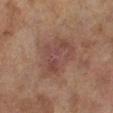Q: Was this lesion biopsied?
A: imaged on a skin check; not biopsied
Q: What is the anatomic site?
A: the left lower leg
Q: How large is the lesion?
A: ≈6.5 mm
Q: Who is the patient?
A: female, in their 60s
Q: What lighting was used for the tile?
A: cross-polarized illumination
Q: Automated lesion metrics?
A: a lesion area of about 18 mm², an outline eccentricity of about 0.75 (0 = round, 1 = elongated), and a symmetry-axis asymmetry near 0.25; a classifier nevus-likeness of about 15/100 and a detector confidence of about 100 out of 100 that the crop contains a lesion
Q: How was this image acquired?
A: ~15 mm tile from a whole-body skin photo